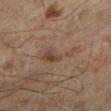<case>
  <biopsy_status>not biopsied; imaged during a skin examination</biopsy_status>
  <lesion_size>
    <long_diameter_mm_approx>5.0</long_diameter_mm_approx>
  </lesion_size>
  <lighting>cross-polarized</lighting>
  <patient>
    <sex>male</sex>
    <age_approx>60</age_approx>
  </patient>
  <site>left lower leg</site>
  <image>
    <source>total-body photography crop</source>
    <field_of_view_mm>15</field_of_view_mm>
  </image>
</case>